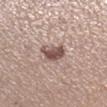follow-up — catalogued during a skin exam; not biopsied | patient — female, aged 33–37 | automated metrics — an area of roughly 5.5 mm², a shape eccentricity near 0.65, and a shape-asymmetry score of about 0.3 (0 = symmetric) | acquisition — ~15 mm crop, total-body skin-cancer survey | body site — the leg | size — about 3 mm.